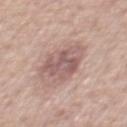Q: Was this lesion biopsied?
A: imaged on a skin check; not biopsied
Q: What kind of image is this?
A: ~15 mm crop, total-body skin-cancer survey
Q: Where on the body is the lesion?
A: the chest
Q: What are the patient's age and sex?
A: male, aged approximately 50
Q: Illumination type?
A: white-light
Q: What did automated image analysis measure?
A: a border-irregularity index near 4/10; a classifier nevus-likeness of about 0/100
Q: How large is the lesion?
A: ≈5 mm